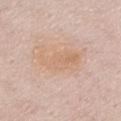Cropped from a total-body skin-imaging series; the visible field is about 15 mm. A female patient, in their 70s. Imaged with white-light lighting. Longest diameter approximately 5.5 mm. The lesion is on the chest. The lesion-visualizer software estimated a footprint of about 13 mm² and two-axis asymmetry of about 0.35. The analysis additionally found a lesion color around L≈67 a*≈18 b*≈32 in CIELAB, a lesion–skin lightness drop of about 6, and a normalized lesion–skin contrast near 5.5.